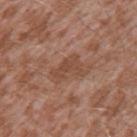Notes:
– biopsy status — no biopsy performed (imaged during a skin exam)
– imaging modality — ~15 mm tile from a whole-body skin photo
– patient — male, approximately 45 years of age
– body site — the left upper arm